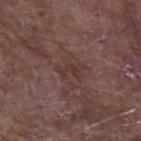The lesion was photographed on a routine skin check and not biopsied; there is no pathology result.
The lesion-visualizer software estimated a lesion area of about 4 mm², an eccentricity of roughly 0.85, and a symmetry-axis asymmetry near 0.55. The software also gave border irregularity of about 7 on a 0–10 scale and a within-lesion color-variation index near 0/10. It also reported an automated nevus-likeness rating near 0 out of 100 and a detector confidence of about 95 out of 100 that the crop contains a lesion.
From the left upper arm.
The recorded lesion diameter is about 3.5 mm.
A male subject in their mid- to late 60s.
A 15 mm crop from a total-body photograph taken for skin-cancer surveillance.